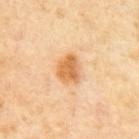notes: no biopsy performed (imaged during a skin exam); patient: male, in their mid- to late 60s; size: ≈3.5 mm; lighting: cross-polarized; image source: total-body-photography crop, ~15 mm field of view.